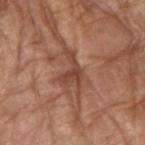Assessment:
Imaged during a routine full-body skin examination; the lesion was not biopsied and no histopathology is available.
Context:
Imaged with white-light lighting. About 5 mm across. The subject is a female approximately 75 years of age. A 15 mm close-up tile from a total-body photography series done for melanoma screening. On the left forearm.